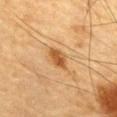{"biopsy_status": "not biopsied; imaged during a skin examination", "site": "chest", "image": {"source": "total-body photography crop", "field_of_view_mm": 15}, "lighting": "cross-polarized", "automated_metrics": {"area_mm2_approx": 4.0, "eccentricity": 0.85, "nevus_likeness_0_100": 85, "lesion_detection_confidence_0_100": 100}, "patient": {"sex": "male", "age_approx": "85+"}, "lesion_size": {"long_diameter_mm_approx": 3.0}}